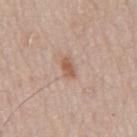diameter: ≈2.5 mm; illumination: white-light illumination; patient: male, in their mid- to late 60s; image source: 15 mm crop, total-body photography; site: the mid back; automated lesion analysis: a border-irregularity index near 2.5/10 and a color-variation rating of about 2/10.